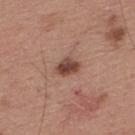Clinical impression: Recorded during total-body skin imaging; not selected for excision or biopsy. Acquisition and patient details: Captured under white-light illumination. A male patient, about 55 years old. A 15 mm crop from a total-body photograph taken for skin-cancer surveillance. From the upper back. Automated tile analysis of the lesion measured an average lesion color of about L≈45 a*≈21 b*≈26 (CIELAB), a lesion–skin lightness drop of about 14, and a normalized lesion–skin contrast near 10. The software also gave a classifier nevus-likeness of about 90/100.